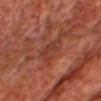notes = catalogued during a skin exam; not biopsied | lesion size = ~4 mm (longest diameter) | illumination = cross-polarized | acquisition = ~15 mm crop, total-body skin-cancer survey | subject = male, about 60 years old | location = the chest.